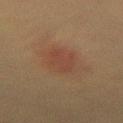Clinical impression: Part of a total-body skin-imaging series; this lesion was reviewed on a skin check and was not flagged for biopsy. Context: The tile uses cross-polarized illumination. On the lower back. A 15 mm crop from a total-body photograph taken for skin-cancer surveillance. Approximately 4 mm at its widest. A female patient, aged 53 to 57.